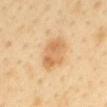<record>
  <biopsy_status>not biopsied; imaged during a skin examination</biopsy_status>
  <image>
    <source>total-body photography crop</source>
    <field_of_view_mm>15</field_of_view_mm>
  </image>
  <automated_metrics>
    <cielab_L>66</cielab_L>
    <cielab_a>19</cielab_a>
    <cielab_b>41</cielab_b>
    <vs_skin_darker_L>10.0</vs_skin_darker_L>
    <vs_skin_contrast_norm>6.5</vs_skin_contrast_norm>
    <border_irregularity_0_10>2.0</border_irregularity_0_10>
    <peripheral_color_asymmetry>1.5</peripheral_color_asymmetry>
    <lesion_detection_confidence_0_100>100</lesion_detection_confidence_0_100>
  </automated_metrics>
  <patient>
    <sex>female</sex>
    <age_approx>50</age_approx>
  </patient>
  <lesion_size>
    <long_diameter_mm_approx>5.0</long_diameter_mm_approx>
  </lesion_size>
  <site>mid back</site>
</record>